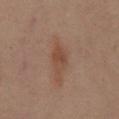site = the abdomen; tile lighting = cross-polarized; lesion size = about 4 mm; image = ~15 mm crop, total-body skin-cancer survey; patient = female, approximately 55 years of age.